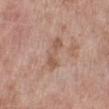Imaged during a routine full-body skin examination; the lesion was not biopsied and no histopathology is available.
A female patient, aged 68 to 72.
On the right lower leg.
Captured under white-light illumination.
A lesion tile, about 15 mm wide, cut from a 3D total-body photograph.